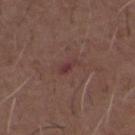Assessment:
The lesion was tiled from a total-body skin photograph and was not biopsied.
Background:
The recorded lesion diameter is about 2.5 mm. The subject is a male roughly 50 years of age. Automated image analysis of the tile measured a lesion color around L≈35 a*≈23 b*≈18 in CIELAB, roughly 7 lightness units darker than nearby skin, and a lesion-to-skin contrast of about 7.5 (normalized; higher = more distinct). Imaged with white-light lighting. A 15 mm close-up tile from a total-body photography series done for melanoma screening. From the chest.